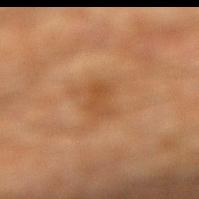Findings:
– notes · catalogued during a skin exam; not biopsied
– lesion diameter · about 3 mm
– TBP lesion metrics · a lesion color around L≈44 a*≈21 b*≈35 in CIELAB and a lesion-to-skin contrast of about 5.5 (normalized; higher = more distinct); border irregularity of about 3.5 on a 0–10 scale, internal color variation of about 2 on a 0–10 scale, and radial color variation of about 0.5; an automated nevus-likeness rating near 5 out of 100 and lesion-presence confidence of about 100/100
– subject · male, aged around 65
– image source · 15 mm crop, total-body photography
– tile lighting · cross-polarized
– location · the right lower leg